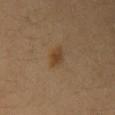biopsy_status: not biopsied; imaged during a skin examination
site: right upper arm
image:
  source: total-body photography crop
  field_of_view_mm: 15
lighting: cross-polarized
lesion_size:
  long_diameter_mm_approx: 2.5
patient:
  sex: female
  age_approx: 35